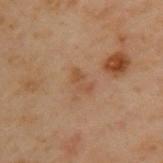biopsy status: no biopsy performed (imaged during a skin exam) | imaging modality: ~15 mm tile from a whole-body skin photo | site: the back | lesion diameter: about 2.5 mm | TBP lesion metrics: a mean CIELAB color near L≈40 a*≈17 b*≈28 and a normalized lesion–skin contrast near 5; a border-irregularity index near 3.5/10 and internal color variation of about 0.5 on a 0–10 scale; a nevus-likeness score of about 0/100 and a lesion-detection confidence of about 100/100 | tile lighting: cross-polarized illumination | subject: male, aged around 55.